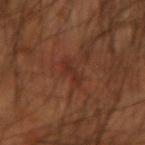| feature | finding |
|---|---|
| follow-up | no biopsy performed (imaged during a skin exam) |
| site | the left forearm |
| subject | male, approximately 55 years of age |
| automated lesion analysis | a mean CIELAB color near L≈27 a*≈21 b*≈25, a lesion–skin lightness drop of about 5, and a normalized border contrast of about 6; a border-irregularity index near 6.5/10, a within-lesion color-variation index near 0/10, and peripheral color asymmetry of about 0 |
| diameter | ~3.5 mm (longest diameter) |
| imaging modality | ~15 mm crop, total-body skin-cancer survey |